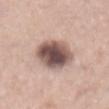{"biopsy_status": "not biopsied; imaged during a skin examination", "image": {"source": "total-body photography crop", "field_of_view_mm": 15}, "site": "mid back", "lesion_size": {"long_diameter_mm_approx": 6.0}, "patient": {"sex": "male", "age_approx": 65}, "lighting": "white-light"}